The patient is a male aged around 65; from the head or neck; a 15 mm crop from a total-body photograph taken for skin-cancer surveillance: 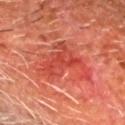Case summary:
- diagnosis · a nodular basal cell carcinoma